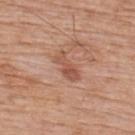Q: Was a biopsy performed?
A: no biopsy performed (imaged during a skin exam)
Q: Illumination type?
A: white-light
Q: Automated lesion metrics?
A: a lesion area of about 5 mm², an eccentricity of roughly 0.9, and a symmetry-axis asymmetry near 0.35; a lesion color around L≈53 a*≈24 b*≈30 in CIELAB and a lesion–skin lightness drop of about 9; border irregularity of about 5 on a 0–10 scale and a within-lesion color-variation index near 2/10; a nevus-likeness score of about 0/100 and a detector confidence of about 100 out of 100 that the crop contains a lesion
Q: How was this image acquired?
A: total-body-photography crop, ~15 mm field of view
Q: What is the anatomic site?
A: the upper back
Q: What is the lesion's diameter?
A: ~4 mm (longest diameter)
Q: Patient demographics?
A: male, in their 60s